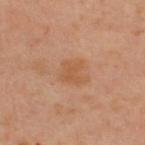workup: catalogued during a skin exam; not biopsied | body site: the back | patient: male, about 45 years old | image source: total-body-photography crop, ~15 mm field of view | tile lighting: cross-polarized illumination.